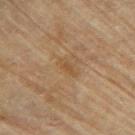The lesion was photographed on a routine skin check and not biopsied; there is no pathology result. Located on the upper back. A female subject about 80 years old. This image is a 15 mm lesion crop taken from a total-body photograph. The recorded lesion diameter is about 2.5 mm.